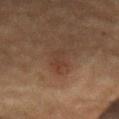Captured during whole-body skin photography for melanoma surveillance; the lesion was not biopsied. A male patient aged 83 or over. An algorithmic analysis of the crop reported an area of roughly 3.5 mm² and two-axis asymmetry of about 0.4. The software also gave a lesion–skin lightness drop of about 5 and a normalized lesion–skin contrast near 5. The software also gave border irregularity of about 4.5 on a 0–10 scale, a color-variation rating of about 0.5/10, and a peripheral color-asymmetry measure near 0. The software also gave a nevus-likeness score of about 25/100 and a detector confidence of about 100 out of 100 that the crop contains a lesion. The lesion is located on the abdomen. This image is a 15 mm lesion crop taken from a total-body photograph.